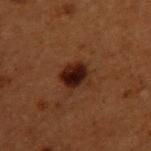  biopsy_status: not biopsied; imaged during a skin examination